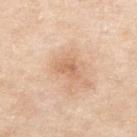Q: Where on the body is the lesion?
A: the right upper arm
Q: Who is the patient?
A: female, aged approximately 70
Q: How was this image acquired?
A: total-body-photography crop, ~15 mm field of view